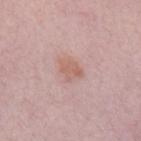workup: imaged on a skin check; not biopsied
anatomic site: the chest
acquisition: 15 mm crop, total-body photography
patient: female, about 40 years old
illumination: white-light illumination
diameter: ~3 mm (longest diameter)